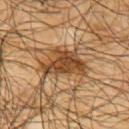Clinical impression:
Captured during whole-body skin photography for melanoma surveillance; the lesion was not biopsied.
Image and clinical context:
A male subject aged approximately 65. The lesion is located on the upper back. A 15 mm crop from a total-body photograph taken for skin-cancer surveillance.